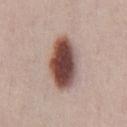The patient is a male about 35 years old.
A 15 mm close-up tile from a total-body photography series done for melanoma screening.
Imaged with white-light lighting.
From the abdomen.
Measured at roughly 6.5 mm in maximum diameter.